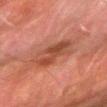Captured during whole-body skin photography for melanoma surveillance; the lesion was not biopsied. Cropped from a whole-body photographic skin survey; the tile spans about 15 mm. About 4.5 mm across. The lesion is located on the right forearm. The subject is a male in their 80s.